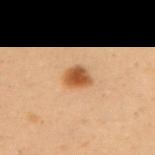Recorded during total-body skin imaging; not selected for excision or biopsy. A lesion tile, about 15 mm wide, cut from a 3D total-body photograph. A female patient, roughly 50 years of age. From the back.